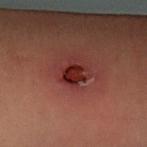<case>
<biopsy_status>not biopsied; imaged during a skin examination</biopsy_status>
<site>left forearm</site>
<image>
  <source>total-body photography crop</source>
  <field_of_view_mm>15</field_of_view_mm>
</image>
<patient>
  <sex>male</sex>
  <age_approx>50</age_approx>
</patient>
</case>